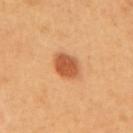No biopsy was performed on this lesion — it was imaged during a full skin examination and was not determined to be concerning. On the upper back. The recorded lesion diameter is about 4 mm. A 15 mm crop from a total-body photograph taken for skin-cancer surveillance. The subject is a female approximately 60 years of age. The lesion-visualizer software estimated an area of roughly 7.5 mm², an outline eccentricity of about 0.7 (0 = round, 1 = elongated), and a shape-asymmetry score of about 0.15 (0 = symmetric). It also reported a nevus-likeness score of about 100/100 and lesion-presence confidence of about 100/100. The tile uses cross-polarized illumination.